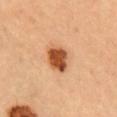notes — total-body-photography surveillance lesion; no biopsy
imaging modality — ~15 mm crop, total-body skin-cancer survey
location — the head or neck
subject — female, in their mid- to late 30s